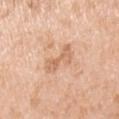Q: Was this lesion biopsied?
A: catalogued during a skin exam; not biopsied
Q: Who is the patient?
A: male, in their 50s
Q: How was the tile lit?
A: white-light
Q: What is the imaging modality?
A: total-body-photography crop, ~15 mm field of view
Q: What is the anatomic site?
A: the right upper arm